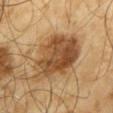Recorded during total-body skin imaging; not selected for excision or biopsy. Located on the mid back. This is a cross-polarized tile. The recorded lesion diameter is about 6.5 mm. A male patient, in their mid-60s. A lesion tile, about 15 mm wide, cut from a 3D total-body photograph.